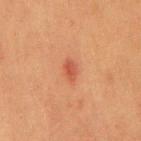biopsy status=total-body-photography surveillance lesion; no biopsy | image source=15 mm crop, total-body photography | patient=male, aged around 40 | diameter=≈2.5 mm | site=the mid back.